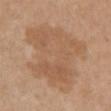Q: Was a biopsy performed?
A: no biopsy performed (imaged during a skin exam)
Q: How was this image acquired?
A: ~15 mm crop, total-body skin-cancer survey
Q: Who is the patient?
A: female, aged around 55
Q: Lesion location?
A: the front of the torso